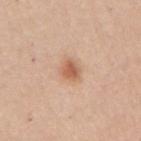Clinical impression: This lesion was catalogued during total-body skin photography and was not selected for biopsy. Image and clinical context: The lesion is located on the arm. The patient is a female aged 63 to 67. Cropped from a whole-body photographic skin survey; the tile spans about 15 mm. Approximately 2.5 mm at its widest.